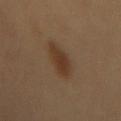Automated image analysis of the tile measured an area of roughly 8.5 mm², a shape eccentricity near 0.8, and a shape-asymmetry score of about 0.25 (0 = symmetric). The analysis additionally found a lesion color around L≈36 a*≈16 b*≈29 in CIELAB. It also reported a detector confidence of about 100 out of 100 that the crop contains a lesion.
Located on the mid back.
This image is a 15 mm lesion crop taken from a total-body photograph.
Approximately 4.5 mm at its widest.
The patient is a female in their 60s.
This is a cross-polarized tile.